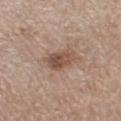Clinical impression:
Imaged during a routine full-body skin examination; the lesion was not biopsied and no histopathology is available.
Image and clinical context:
Cropped from a total-body skin-imaging series; the visible field is about 15 mm. Located on the left thigh. A female subject in their 40s. Captured under white-light illumination.